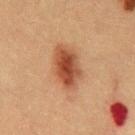<record>
  <biopsy_status>not biopsied; imaged during a skin examination</biopsy_status>
  <site>front of the torso</site>
  <image>
    <source>total-body photography crop</source>
    <field_of_view_mm>15</field_of_view_mm>
  </image>
  <lesion_size>
    <long_diameter_mm_approx>5.5</long_diameter_mm_approx>
  </lesion_size>
  <automated_metrics>
    <area_mm2_approx>13.0</area_mm2_approx>
    <shape_asymmetry>0.2</shape_asymmetry>
  </automated_metrics>
  <patient>
    <sex>male</sex>
    <age_approx>50</age_approx>
  </patient>
  <lighting>cross-polarized</lighting>
</record>